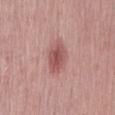{"biopsy_status": "not biopsied; imaged during a skin examination", "site": "mid back", "lesion_size": {"long_diameter_mm_approx": 4.5}, "automated_metrics": {"area_mm2_approx": 8.5, "shape_asymmetry": 0.2, "cielab_L": 54, "cielab_a": 25, "cielab_b": 23, "vs_skin_darker_L": 11.0, "nevus_likeness_0_100": 75, "lesion_detection_confidence_0_100": 100}, "lighting": "white-light", "image": {"source": "total-body photography crop", "field_of_view_mm": 15}, "patient": {"sex": "male", "age_approx": 60}}